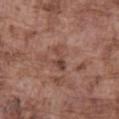Part of a total-body skin-imaging series; this lesion was reviewed on a skin check and was not flagged for biopsy.
An algorithmic analysis of the crop reported an average lesion color of about L≈44 a*≈21 b*≈25 (CIELAB) and about 9 CIELAB-L* units darker than the surrounding skin. The analysis additionally found a border-irregularity rating of about 5/10, a color-variation rating of about 1/10, and peripheral color asymmetry of about 0. And it measured an automated nevus-likeness rating near 0 out of 100 and a detector confidence of about 100 out of 100 that the crop contains a lesion.
The lesion is on the front of the torso.
The patient is a male aged approximately 75.
This is a white-light tile.
Longest diameter approximately 3.5 mm.
This image is a 15 mm lesion crop taken from a total-body photograph.